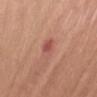Findings:
* body site: the abdomen
* imaging modality: total-body-photography crop, ~15 mm field of view
* patient: female, in their 50s